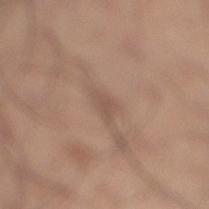Imaged during a routine full-body skin examination; the lesion was not biopsied and no histopathology is available. Approximately 3 mm at its widest. Located on the leg. Automated tile analysis of the lesion measured a border-irregularity index near 4/10 and peripheral color asymmetry of about 0.5. A 15 mm crop from a total-body photograph taken for skin-cancer surveillance. A male subject aged around 35.